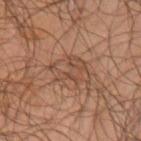The lesion was tiled from a total-body skin photograph and was not biopsied. Cropped from a whole-body photographic skin survey; the tile spans about 15 mm. Imaged with cross-polarized lighting. The subject is a male in their mid-60s. Located on the left upper arm.